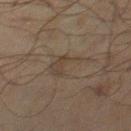Captured during whole-body skin photography for melanoma surveillance; the lesion was not biopsied.
A lesion tile, about 15 mm wide, cut from a 3D total-body photograph.
A male patient, aged 68–72.
On the right thigh.